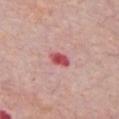- biopsy status: catalogued during a skin exam; not biopsied
- image: ~15 mm crop, total-body skin-cancer survey
- site: the chest
- patient: male, aged approximately 65